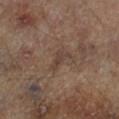Part of a total-body skin-imaging series; this lesion was reviewed on a skin check and was not flagged for biopsy.
On the left lower leg.
A male patient roughly 65 years of age.
Cropped from a whole-body photographic skin survey; the tile spans about 15 mm.